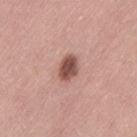{
  "biopsy_status": "not biopsied; imaged during a skin examination",
  "site": "left thigh",
  "patient": {
    "sex": "female",
    "age_approx": 50
  },
  "image": {
    "source": "total-body photography crop",
    "field_of_view_mm": 15
  }
}